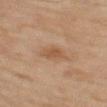Notes:
• biopsy status: catalogued during a skin exam; not biopsied
• illumination: cross-polarized
• acquisition: 15 mm crop, total-body photography
• site: the leg
• patient: female, aged 58 to 62